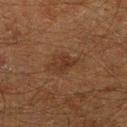Notes:
- biopsy status: imaged on a skin check; not biopsied
- anatomic site: the right lower leg
- lesion diameter: about 3.5 mm
- image-analysis metrics: a mean CIELAB color near L≈26 a*≈15 b*≈23, roughly 5 lightness units darker than nearby skin, and a normalized lesion–skin contrast near 5.5; a border-irregularity index near 3/10 and internal color variation of about 2 on a 0–10 scale; a nevus-likeness score of about 60/100
- patient: male, aged approximately 60
- imaging modality: ~15 mm crop, total-body skin-cancer survey